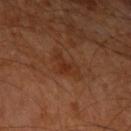biopsy_status: not biopsied; imaged during a skin examination
patient:
  sex: male
  age_approx: 60
image:
  source: total-body photography crop
  field_of_view_mm: 15
site: left upper arm